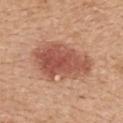No biopsy was performed on this lesion — it was imaged during a full skin examination and was not determined to be concerning. An algorithmic analysis of the crop reported an area of roughly 26 mm², an eccentricity of roughly 0.85, and a symmetry-axis asymmetry near 0.2. The analysis additionally found an average lesion color of about L≈54 a*≈25 b*≈30 (CIELAB), about 13 CIELAB-L* units darker than the surrounding skin, and a lesion-to-skin contrast of about 8.5 (normalized; higher = more distinct). The analysis additionally found a classifier nevus-likeness of about 95/100 and a lesion-detection confidence of about 100/100. The lesion is located on the upper back. This image is a 15 mm lesion crop taken from a total-body photograph. A female patient, about 55 years old. The tile uses white-light illumination. Approximately 8 mm at its widest.